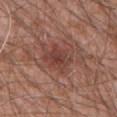The lesion was tiled from a total-body skin photograph and was not biopsied. Imaged with white-light lighting. Longest diameter approximately 3.5 mm. A 15 mm close-up extracted from a 3D total-body photography capture. A male patient, approximately 60 years of age. The lesion-visualizer software estimated a lesion color around L≈41 a*≈23 b*≈25 in CIELAB, roughly 8 lightness units darker than nearby skin, and a normalized lesion–skin contrast near 6.5. It also reported a classifier nevus-likeness of about 35/100 and a lesion-detection confidence of about 100/100. The lesion is on the lower back.